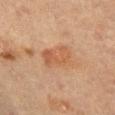Q: Was this lesion biopsied?
A: catalogued during a skin exam; not biopsied
Q: Patient demographics?
A: female, aged approximately 65
Q: What is the anatomic site?
A: the left thigh
Q: Lesion size?
A: ≈4 mm
Q: Automated lesion metrics?
A: a nevus-likeness score of about 5/100 and lesion-presence confidence of about 100/100
Q: What is the imaging modality?
A: ~15 mm tile from a whole-body skin photo
Q: How was the tile lit?
A: cross-polarized illumination